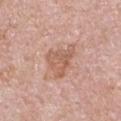Notes:
– biopsy status — catalogued during a skin exam; not biopsied
– patient — male, aged around 55
– image-analysis metrics — an area of roughly 10 mm², an eccentricity of roughly 0.6, and two-axis asymmetry of about 0.45; a border-irregularity index near 4.5/10, a within-lesion color-variation index near 3.5/10, and peripheral color asymmetry of about 1
– lesion diameter — ≈4.5 mm
– image — ~15 mm tile from a whole-body skin photo
– body site — the chest